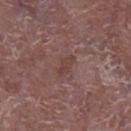Notes:
– follow-up · imaged on a skin check; not biopsied